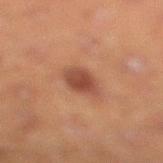Recorded during total-body skin imaging; not selected for excision or biopsy. Located on the right lower leg. The subject is a male approximately 45 years of age. Automated image analysis of the tile measured a classifier nevus-likeness of about 90/100 and a lesion-detection confidence of about 100/100. Longest diameter approximately 3 mm. Imaged with cross-polarized lighting. A lesion tile, about 15 mm wide, cut from a 3D total-body photograph.